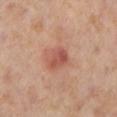<case>
<patient>
  <sex>female</sex>
  <age_approx>55</age_approx>
</patient>
<lesion_size>
  <long_diameter_mm_approx>3.0</long_diameter_mm_approx>
</lesion_size>
<image>
  <source>total-body photography crop</source>
  <field_of_view_mm>15</field_of_view_mm>
</image>
<site>left lower leg</site>
<lighting>cross-polarized</lighting>
</case>